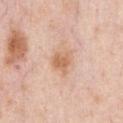Clinical impression: Captured during whole-body skin photography for melanoma surveillance; the lesion was not biopsied. Background: A male patient, approximately 50 years of age. This is a white-light tile. A 15 mm close-up extracted from a 3D total-body photography capture. On the chest. Approximately 2.5 mm at its widest.